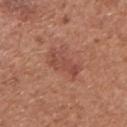<lesion>
<biopsy_status>not biopsied; imaged during a skin examination</biopsy_status>
<image>
  <source>total-body photography crop</source>
  <field_of_view_mm>15</field_of_view_mm>
</image>
<site>chest</site>
<patient>
  <sex>male</sex>
  <age_approx>75</age_approx>
</patient>
<lighting>white-light</lighting>
</lesion>